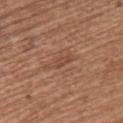<lesion>
<site>back</site>
<patient>
  <sex>female</sex>
  <age_approx>60</age_approx>
</patient>
<automated_metrics>
  <area_mm2_approx>3.5</area_mm2_approx>
  <eccentricity>0.95</eccentricity>
  <shape_asymmetry>0.35</shape_asymmetry>
  <cielab_L>47</cielab_L>
  <cielab_a>22</cielab_a>
  <cielab_b>28</cielab_b>
  <vs_skin_contrast_norm>5.5</vs_skin_contrast_norm>
  <border_irregularity_0_10>5.0</border_irregularity_0_10>
  <color_variation_0_10>0.0</color_variation_0_10>
  <peripheral_color_asymmetry>0.0</peripheral_color_asymmetry>
  <nevus_likeness_0_100>0</nevus_likeness_0_100>
</automated_metrics>
<lesion_size>
  <long_diameter_mm_approx>3.5</long_diameter_mm_approx>
</lesion_size>
<image>
  <source>total-body photography crop</source>
  <field_of_view_mm>15</field_of_view_mm>
</image>
</lesion>